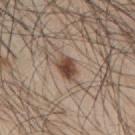Case summary:
* notes · no biopsy performed (imaged during a skin exam)
* subject · male, aged 43–47
* image · total-body-photography crop, ~15 mm field of view
* location · the mid back